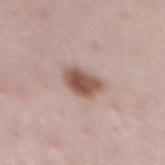{
  "biopsy_status": "not biopsied; imaged during a skin examination",
  "site": "mid back",
  "lighting": "white-light",
  "lesion_size": {
    "long_diameter_mm_approx": 3.5
  },
  "image": {
    "source": "total-body photography crop",
    "field_of_view_mm": 15
  },
  "automated_metrics": {
    "area_mm2_approx": 8.0,
    "eccentricity": 0.7,
    "shape_asymmetry": 0.25,
    "cielab_L": 53,
    "cielab_a": 20,
    "cielab_b": 26,
    "vs_skin_contrast_norm": 10.5,
    "nevus_likeness_0_100": 95,
    "lesion_detection_confidence_0_100": 100
  },
  "patient": {
    "sex": "female",
    "age_approx": 50
  }
}